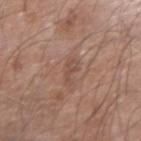| feature | finding |
|---|---|
| workup | total-body-photography surveillance lesion; no biopsy |
| diameter | about 3 mm |
| body site | the left forearm |
| patient | male, aged approximately 70 |
| imaging modality | ~15 mm crop, total-body skin-cancer survey |
| automated metrics | a border-irregularity index near 5/10, a color-variation rating of about 1.5/10, and a peripheral color-asymmetry measure near 0.5 |